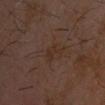{
  "biopsy_status": "not biopsied; imaged during a skin examination",
  "lesion_size": {
    "long_diameter_mm_approx": 3.0
  },
  "site": "head or neck",
  "image": {
    "source": "total-body photography crop",
    "field_of_view_mm": 15
  },
  "lighting": "cross-polarized",
  "automated_metrics": {
    "cielab_L": 27,
    "cielab_a": 15,
    "cielab_b": 22,
    "vs_skin_darker_L": 4.0,
    "vs_skin_contrast_norm": 5.5,
    "border_irregularity_0_10": 6.0,
    "color_variation_0_10": 1.5,
    "peripheral_color_asymmetry": 0.5,
    "nevus_likeness_0_100": 0
  },
  "patient": {
    "sex": "male",
    "age_approx": 70
  }
}